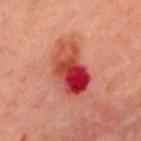Clinical impression: The lesion was photographed on a routine skin check and not biopsied; there is no pathology result. Clinical summary: Cropped from a whole-body photographic skin survey; the tile spans about 15 mm. Located on the upper back. Automated tile analysis of the lesion measured border irregularity of about 2.5 on a 0–10 scale, a within-lesion color-variation index near 10/10, and a peripheral color-asymmetry measure near 7.5. The software also gave a nevus-likeness score of about 0/100 and a lesion-detection confidence of about 100/100. A male patient aged 48–52.